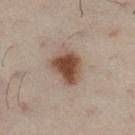Q: Is there a histopathology result?
A: no biopsy performed (imaged during a skin exam)
Q: Where on the body is the lesion?
A: the left leg
Q: What are the patient's age and sex?
A: female, aged 28–32
Q: Automated lesion metrics?
A: an outline eccentricity of about 0.65 (0 = round, 1 = elongated) and two-axis asymmetry of about 0.25; a border-irregularity index near 2.5/10, a within-lesion color-variation index near 3.5/10, and a peripheral color-asymmetry measure near 1; a nevus-likeness score of about 100/100
Q: How was this image acquired?
A: 15 mm crop, total-body photography
Q: What is the lesion's diameter?
A: ~4 mm (longest diameter)
Q: How was the tile lit?
A: cross-polarized illumination